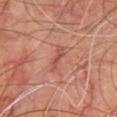No biopsy was performed on this lesion — it was imaged during a full skin examination and was not determined to be concerning.
A male patient in their 70s.
Located on the chest.
An algorithmic analysis of the crop reported border irregularity of about 4 on a 0–10 scale, a color-variation rating of about 1/10, and peripheral color asymmetry of about 0.5. The analysis additionally found a classifier nevus-likeness of about 0/100 and a lesion-detection confidence of about 95/100.
The lesion's longest dimension is about 3.5 mm.
The tile uses cross-polarized illumination.
A 15 mm close-up extracted from a 3D total-body photography capture.